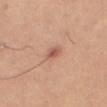{
  "biopsy_status": "not biopsied; imaged during a skin examination",
  "patient": {
    "sex": "female",
    "age_approx": 40
  },
  "automated_metrics": {
    "area_mm2_approx": 6.5,
    "eccentricity": 0.85,
    "nevus_likeness_0_100": 25
  },
  "lighting": "cross-polarized",
  "image": {
    "source": "total-body photography crop",
    "field_of_view_mm": 15
  },
  "site": "right thigh",
  "lesion_size": {
    "long_diameter_mm_approx": 3.5
  }
}